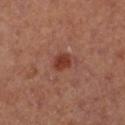Context:
Located on the left lower leg. A female patient. The tile uses cross-polarized illumination. The recorded lesion diameter is about 2.5 mm. A 15 mm close-up tile from a total-body photography series done for melanoma screening.